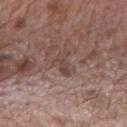Clinical impression: The lesion was tiled from a total-body skin photograph and was not biopsied. Context: A region of skin cropped from a whole-body photographic capture, roughly 15 mm wide. The lesion-visualizer software estimated an area of roughly 4.5 mm², a shape eccentricity near 0.75, and two-axis asymmetry of about 0.55. And it measured a within-lesion color-variation index near 1.5/10 and a peripheral color-asymmetry measure near 0. The lesion's longest dimension is about 3 mm. This is a white-light tile. A male subject, approximately 70 years of age. The lesion is located on the mid back.